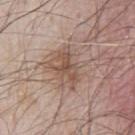Case summary:
• workup: no biopsy performed (imaged during a skin exam)